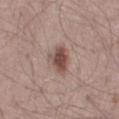image-analysis metrics: a shape eccentricity near 0.7 and a symmetry-axis asymmetry near 0.25; a lesion color around L≈48 a*≈19 b*≈23 in CIELAB, roughly 13 lightness units darker than nearby skin, and a normalized border contrast of about 9.5; a border-irregularity index near 2.5/10, a within-lesion color-variation index near 3/10, and peripheral color asymmetry of about 1
lesion diameter: ~3.5 mm (longest diameter)
body site: the left thigh
patient: male, in their mid-40s
image source: 15 mm crop, total-body photography